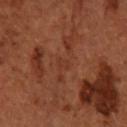Case summary:
* workup · total-body-photography surveillance lesion; no biopsy
* acquisition · 15 mm crop, total-body photography
* TBP lesion metrics · a border-irregularity index near 3/10, a within-lesion color-variation index near 0/10, and peripheral color asymmetry of about 0
* location · the right forearm
* lesion size · ~1.5 mm (longest diameter)
* tile lighting · cross-polarized
* subject · female, in their mid- to late 60s